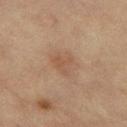A close-up tile cropped from a whole-body skin photograph, about 15 mm across.
The total-body-photography lesion software estimated a lesion area of about 8 mm² and a symmetry-axis asymmetry near 0.25. The analysis additionally found a nevus-likeness score of about 5/100 and a detector confidence of about 100 out of 100 that the crop contains a lesion.
Measured at roughly 3.5 mm in maximum diameter.
The lesion is on the leg.
The patient is a female aged around 55.
Imaged with cross-polarized lighting.